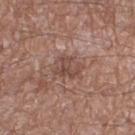About 3 mm across. Imaged with white-light lighting. The patient is a male in their mid-60s. From the right thigh. A 15 mm close-up tile from a total-body photography series done for melanoma screening. Automated image analysis of the tile measured a border-irregularity rating of about 3/10, a color-variation rating of about 4/10, and a peripheral color-asymmetry measure near 1.5. The analysis additionally found a nevus-likeness score of about 0/100 and a lesion-detection confidence of about 95/100.